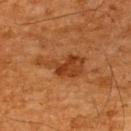Part of a total-body skin-imaging series; this lesion was reviewed on a skin check and was not flagged for biopsy. From the upper back. A male subject roughly 65 years of age. A 15 mm close-up tile from a total-body photography series done for melanoma screening.